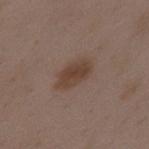Impression: This lesion was catalogued during total-body skin photography and was not selected for biopsy. Acquisition and patient details: Captured under white-light illumination. Cropped from a total-body skin-imaging series; the visible field is about 15 mm. A female patient, aged around 35. On the mid back.